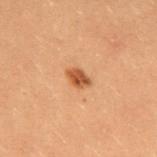Findings:
- follow-up · imaged on a skin check; not biopsied
- image source · ~15 mm tile from a whole-body skin photo
- subject · female, in their 20s
- anatomic site · the upper back